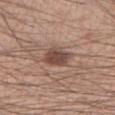biopsy status: imaged on a skin check; not biopsied
imaging modality: 15 mm crop, total-body photography
subject: male, aged 33–37
body site: the right forearm
automated lesion analysis: a lesion area of about 7 mm², an eccentricity of roughly 0.35, and a symmetry-axis asymmetry near 0.15; a lesion color around L≈46 a*≈19 b*≈24 in CIELAB and a lesion-to-skin contrast of about 9 (normalized; higher = more distinct)
lesion size: ~3 mm (longest diameter)
illumination: white-light